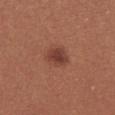This lesion was catalogued during total-body skin photography and was not selected for biopsy. A 15 mm crop from a total-body photograph taken for skin-cancer surveillance. A female patient, approximately 25 years of age. Captured under white-light illumination. Located on the abdomen.